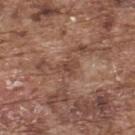Findings:
• notes — imaged on a skin check; not biopsied
• image — ~15 mm crop, total-body skin-cancer survey
• automated metrics — an area of roughly 3.5 mm² and a symmetry-axis asymmetry near 0.35; a mean CIELAB color near L≈45 a*≈20 b*≈26 and a normalized lesion–skin contrast near 6; a classifier nevus-likeness of about 0/100
• lesion size — about 2.5 mm
• lighting — white-light
• subject — male, approximately 75 years of age
• location — the back